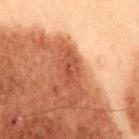Q: Is there a histopathology result?
A: total-body-photography surveillance lesion; no biopsy
Q: How was this image acquired?
A: ~15 mm tile from a whole-body skin photo
Q: What lighting was used for the tile?
A: cross-polarized illumination
Q: What did automated image analysis measure?
A: a border-irregularity rating of about 3.5/10, a color-variation rating of about 2.5/10, and peripheral color asymmetry of about 0.5
Q: Lesion location?
A: the lower back
Q: Patient demographics?
A: male, in their mid-50s
Q: How large is the lesion?
A: about 3.5 mm